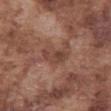Imaged during a routine full-body skin examination; the lesion was not biopsied and no histopathology is available. Cropped from a total-body skin-imaging series; the visible field is about 15 mm. The patient is a male about 75 years old. The lesion is located on the abdomen.